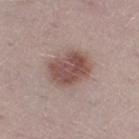Recorded during total-body skin imaging; not selected for excision or biopsy. Imaged with white-light lighting. A male patient aged around 30. Cropped from a whole-body photographic skin survey; the tile spans about 15 mm.